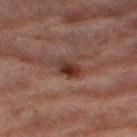Part of a total-body skin-imaging series; this lesion was reviewed on a skin check and was not flagged for biopsy. A female subject aged 68–72. Longest diameter approximately 3 mm. The tile uses cross-polarized illumination. A 15 mm close-up tile from a total-body photography series done for melanoma screening. Automated tile analysis of the lesion measured a footprint of about 4 mm², an outline eccentricity of about 0.8 (0 = round, 1 = elongated), and a shape-asymmetry score of about 0.2 (0 = symmetric). The software also gave a mean CIELAB color near L≈27 a*≈20 b*≈22, roughly 10 lightness units darker than nearby skin, and a normalized border contrast of about 10.5. On the right thigh.